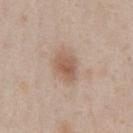follow-up: no biopsy performed (imaged during a skin exam); anatomic site: the chest; acquisition: total-body-photography crop, ~15 mm field of view; patient: male, aged around 60.